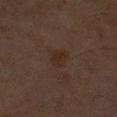{"biopsy_status": "not biopsied; imaged during a skin examination", "patient": {"sex": "male", "age_approx": 60}, "lighting": "cross-polarized", "automated_metrics": {"area_mm2_approx": 5.0, "eccentricity": 0.35, "shape_asymmetry": 0.35, "cielab_L": 24, "cielab_a": 13, "cielab_b": 21, "vs_skin_contrast_norm": 6.0, "border_irregularity_0_10": 3.0, "color_variation_0_10": 2.5, "peripheral_color_asymmetry": 1.0}, "site": "left upper arm", "image": {"source": "total-body photography crop", "field_of_view_mm": 15}, "lesion_size": {"long_diameter_mm_approx": 2.5}}